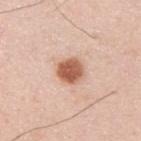The lesion was photographed on a routine skin check and not biopsied; there is no pathology result.
The lesion's longest dimension is about 3 mm.
A male subject aged 33 to 37.
A close-up tile cropped from a whole-body skin photograph, about 15 mm across.
Located on the upper back.
This is a white-light tile.